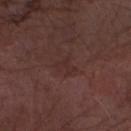Assessment:
No biopsy was performed on this lesion — it was imaged during a full skin examination and was not determined to be concerning.
Acquisition and patient details:
The lesion's longest dimension is about 2.5 mm. This is a white-light tile. A close-up tile cropped from a whole-body skin photograph, about 15 mm across. A male subject in their mid-70s. Automated image analysis of the tile measured an area of roughly 3 mm² and two-axis asymmetry of about 0.55. It also reported an average lesion color of about L≈28 a*≈19 b*≈19 (CIELAB). And it measured border irregularity of about 7.5 on a 0–10 scale and a color-variation rating of about 0/10. And it measured a classifier nevus-likeness of about 0/100 and a lesion-detection confidence of about 100/100. The lesion is located on the right forearm.